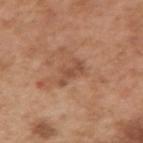Recorded during total-body skin imaging; not selected for excision or biopsy.
A close-up tile cropped from a whole-body skin photograph, about 15 mm across.
On the right upper arm.
The lesion's longest dimension is about 3.5 mm.
The patient is a male aged around 55.
This is a white-light tile.